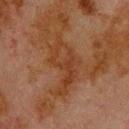Clinical impression:
Captured during whole-body skin photography for melanoma surveillance; the lesion was not biopsied.
Image and clinical context:
Imaged with cross-polarized lighting. Cropped from a whole-body photographic skin survey; the tile spans about 15 mm. The lesion is located on the back. The lesion's longest dimension is about 7 mm. A male subject, aged 78 to 82.